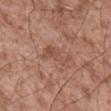illumination: white-light illumination; lesion size: ≈4.5 mm; site: the mid back; imaging modality: ~15 mm tile from a whole-body skin photo; subject: male, aged 53 to 57.